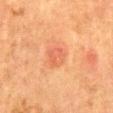Q: Is there a histopathology result?
A: no biopsy performed (imaged during a skin exam)
Q: Who is the patient?
A: female, in their 50s
Q: Lesion location?
A: the abdomen
Q: How large is the lesion?
A: about 3 mm
Q: How was the tile lit?
A: cross-polarized illumination
Q: What did automated image analysis measure?
A: an eccentricity of roughly 0.65 and two-axis asymmetry of about 0.15; border irregularity of about 1.5 on a 0–10 scale, internal color variation of about 3 on a 0–10 scale, and peripheral color asymmetry of about 1
Q: How was this image acquired?
A: total-body-photography crop, ~15 mm field of view